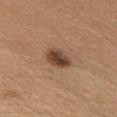Captured during whole-body skin photography for melanoma surveillance; the lesion was not biopsied. Imaged with white-light lighting. Located on the chest. A female subject approximately 45 years of age. A 15 mm close-up tile from a total-body photography series done for melanoma screening. Measured at roughly 3.5 mm in maximum diameter.